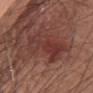workup: no biopsy performed (imaged during a skin exam) | image source: ~15 mm tile from a whole-body skin photo | lesion size: ~6 mm (longest diameter) | illumination: white-light | location: the upper back | subject: male, about 70 years old.